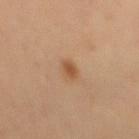Case summary:
* workup: total-body-photography surveillance lesion; no biopsy
* illumination: cross-polarized
* image source: ~15 mm crop, total-body skin-cancer survey
* body site: the mid back
* subject: male, aged 58 to 62